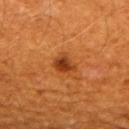Case summary:
* follow-up · no biopsy performed (imaged during a skin exam)
* imaging modality · 15 mm crop, total-body photography
* body site · the mid back
* lesion diameter · about 2.5 mm
* image-analysis metrics · a lesion area of about 4.5 mm², an outline eccentricity of about 0.6 (0 = round, 1 = elongated), and a shape-asymmetry score of about 0.2 (0 = symmetric); a mean CIELAB color near L≈38 a*≈29 b*≈40 and a lesion-to-skin contrast of about 9.5 (normalized; higher = more distinct); a classifier nevus-likeness of about 100/100 and a lesion-detection confidence of about 100/100
* subject · male, aged 58 to 62